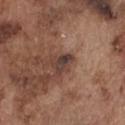Q: Was a biopsy performed?
A: total-body-photography surveillance lesion; no biopsy
Q: What is the anatomic site?
A: the chest
Q: What kind of image is this?
A: 15 mm crop, total-body photography
Q: What are the patient's age and sex?
A: male, about 75 years old
Q: Illumination type?
A: white-light illumination